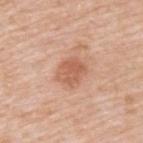{
  "biopsy_status": "not biopsied; imaged during a skin examination",
  "lighting": "white-light",
  "patient": {
    "sex": "male",
    "age_approx": 80
  },
  "image": {
    "source": "total-body photography crop",
    "field_of_view_mm": 15
  },
  "site": "upper back",
  "lesion_size": {
    "long_diameter_mm_approx": 3.5
  }
}